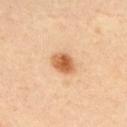The lesion was tiled from a total-body skin photograph and was not biopsied. About 3 mm across. A roughly 15 mm field-of-view crop from a total-body skin photograph. The lesion is located on the upper back. The subject is a male in their mid-30s.